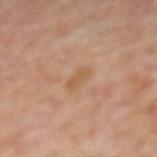This lesion was catalogued during total-body skin photography and was not selected for biopsy.
Cropped from a whole-body photographic skin survey; the tile spans about 15 mm.
Imaged with cross-polarized lighting.
The lesion-visualizer software estimated a footprint of about 3 mm², a shape eccentricity near 0.9, and a symmetry-axis asymmetry near 0.4. The software also gave an average lesion color of about L≈53 a*≈20 b*≈33 (CIELAB), roughly 7 lightness units darker than nearby skin, and a normalized lesion–skin contrast near 6. The analysis additionally found a border-irregularity rating of about 4/10, internal color variation of about 0 on a 0–10 scale, and a peripheral color-asymmetry measure near 0.
On the mid back.
The recorded lesion diameter is about 2.5 mm.
A male patient, aged approximately 70.